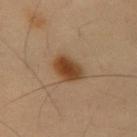Case summary:
- notes — total-body-photography surveillance lesion; no biopsy
- lighting — cross-polarized
- TBP lesion metrics — an eccentricity of roughly 0.65 and a symmetry-axis asymmetry near 0.2
- patient — male, aged 53–57
- anatomic site — the mid back
- image source — ~15 mm tile from a whole-body skin photo